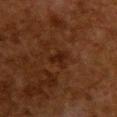{"biopsy_status": "not biopsied; imaged during a skin examination", "site": "back", "image": {"source": "total-body photography crop", "field_of_view_mm": 15}, "lesion_size": {"long_diameter_mm_approx": 2.5}, "patient": {"sex": "female", "age_approx": 50}}